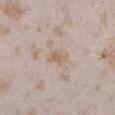The lesion was tiled from a total-body skin photograph and was not biopsied. The subject is a female approximately 25 years of age. Located on the left lower leg. A 15 mm crop from a total-body photograph taken for skin-cancer surveillance.